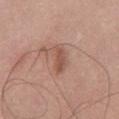{"biopsy_status": "not biopsied; imaged during a skin examination", "site": "front of the torso", "patient": {"sex": "male", "age_approx": 55}, "image": {"source": "total-body photography crop", "field_of_view_mm": 15}}